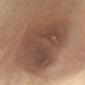follow-up = total-body-photography surveillance lesion; no biopsy
subject = male, aged around 70
anatomic site = the abdomen
lighting = cross-polarized
size = ~10 mm (longest diameter)
image = ~15 mm crop, total-body skin-cancer survey